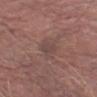Q: Is there a histopathology result?
A: no biopsy performed (imaged during a skin exam)
Q: What kind of image is this?
A: 15 mm crop, total-body photography
Q: What did automated image analysis measure?
A: an eccentricity of roughly 0.7 and a shape-asymmetry score of about 0.2 (0 = symmetric); an average lesion color of about L≈45 a*≈17 b*≈20 (CIELAB), roughly 5 lightness units darker than nearby skin, and a lesion-to-skin contrast of about 5 (normalized; higher = more distinct); a border-irregularity rating of about 2.5/10, internal color variation of about 1.5 on a 0–10 scale, and radial color variation of about 0.5; a classifier nevus-likeness of about 0/100 and a lesion-detection confidence of about 70/100
Q: Where on the body is the lesion?
A: the right forearm
Q: What lighting was used for the tile?
A: white-light illumination
Q: What are the patient's age and sex?
A: male, in their mid-70s
Q: How large is the lesion?
A: ~3.5 mm (longest diameter)